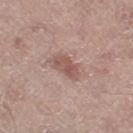  lesion_size:
    long_diameter_mm_approx: 3.5
  image:
    source: total-body photography crop
    field_of_view_mm: 15
  patient:
    sex: male
    age_approx: 65
  site: leg